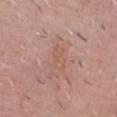<tbp_lesion>
  <biopsy_status>not biopsied; imaged during a skin examination</biopsy_status>
  <lighting>white-light</lighting>
  <image>
    <source>total-body photography crop</source>
    <field_of_view_mm>15</field_of_view_mm>
  </image>
  <patient>
    <sex>male</sex>
    <age_approx>55</age_approx>
  </patient>
  <lesion_size>
    <long_diameter_mm_approx>3.0</long_diameter_mm_approx>
  </lesion_size>
  <site>chest</site>
</tbp_lesion>